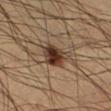biopsy_status: not biopsied; imaged during a skin examination